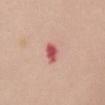Q: Is there a histopathology result?
A: no biopsy performed (imaged during a skin exam)
Q: What is the anatomic site?
A: the chest
Q: What kind of image is this?
A: ~15 mm tile from a whole-body skin photo
Q: Who is the patient?
A: female, roughly 50 years of age
Q: What lighting was used for the tile?
A: white-light illumination